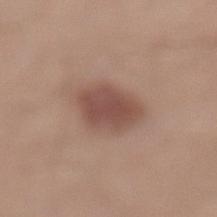No biopsy was performed on this lesion — it was imaged during a full skin examination and was not determined to be concerning. A male subject in their 30s. A close-up tile cropped from a whole-body skin photograph, about 15 mm across. About 4.5 mm across. The tile uses white-light illumination. The lesion is located on the leg.